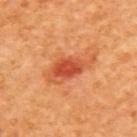Imaged during a routine full-body skin examination; the lesion was not biopsied and no histopathology is available. This image is a 15 mm lesion crop taken from a total-body photograph. A female patient, about 40 years old. The lesion is on the back.